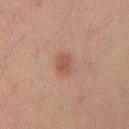No biopsy was performed on this lesion — it was imaged during a full skin examination and was not determined to be concerning. Automated image analysis of the tile measured lesion-presence confidence of about 100/100. The patient is a female aged approximately 25. On the right upper arm. Measured at roughly 2.5 mm in maximum diameter. A 15 mm close-up extracted from a 3D total-body photography capture.